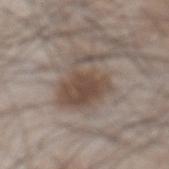Imaged during a routine full-body skin examination; the lesion was not biopsied and no histopathology is available. The tile uses white-light illumination. A lesion tile, about 15 mm wide, cut from a 3D total-body photograph. The lesion's longest dimension is about 6 mm. A male subject in their mid- to late 50s. An algorithmic analysis of the crop reported a shape eccentricity near 0.5 and a shape-asymmetry score of about 0.5 (0 = symmetric). The software also gave a lesion-to-skin contrast of about 8 (normalized; higher = more distinct). The software also gave a border-irregularity rating of about 7.5/10 and internal color variation of about 3.5 on a 0–10 scale. It also reported an automated nevus-likeness rating near 90 out of 100 and lesion-presence confidence of about 100/100. From the abdomen.